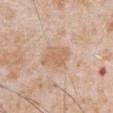This lesion was catalogued during total-body skin photography and was not selected for biopsy.
The lesion is located on the abdomen.
This is a white-light tile.
A 15 mm crop from a total-body photograph taken for skin-cancer surveillance.
The subject is a male aged 63 to 67.
The recorded lesion diameter is about 4 mm.